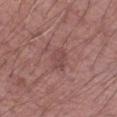workup — total-body-photography surveillance lesion; no biopsy | lighting — white-light | location — the left forearm | lesion diameter — ≈3 mm | acquisition — ~15 mm tile from a whole-body skin photo | subject — male, aged 68–72.